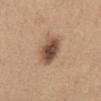A 15 mm close-up extracted from a 3D total-body photography capture. Captured under white-light illumination. A female subject about 45 years old. Located on the front of the torso. The lesion's longest dimension is about 4.5 mm.